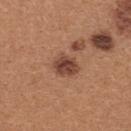The lesion was photographed on a routine skin check and not biopsied; there is no pathology result.
Approximately 3 mm at its widest.
A roughly 15 mm field-of-view crop from a total-body skin photograph.
The patient is a female approximately 40 years of age.
The lesion is located on the upper back.